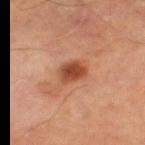Assessment:
Recorded during total-body skin imaging; not selected for excision or biopsy.
Clinical summary:
This image is a 15 mm lesion crop taken from a total-body photograph. On the left lower leg. A male subject aged 68–72.